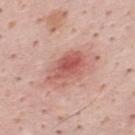Clinical impression: The lesion was tiled from a total-body skin photograph and was not biopsied. Clinical summary: Cropped from a total-body skin-imaging series; the visible field is about 15 mm. Imaged with white-light lighting. A male subject aged around 35. The lesion is located on the mid back. Automated tile analysis of the lesion measured a lesion–skin lightness drop of about 11 and a lesion-to-skin contrast of about 7.5 (normalized; higher = more distinct).